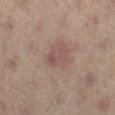This lesion was catalogued during total-body skin photography and was not selected for biopsy. The lesion is located on the left leg. Cropped from a total-body skin-imaging series; the visible field is about 15 mm. A female subject approximately 40 years of age.